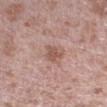workup: no biopsy performed (imaged during a skin exam) | size: ≈2.5 mm | location: the right upper arm | acquisition: ~15 mm crop, total-body skin-cancer survey | automated metrics: a mean CIELAB color near L≈54 a*≈20 b*≈25 and a lesion–skin lightness drop of about 9; a border-irregularity rating of about 3.5/10, a color-variation rating of about 3/10, and a peripheral color-asymmetry measure near 1 | subject: male, aged around 40 | lighting: white-light.